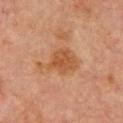Clinical impression:
Captured during whole-body skin photography for melanoma surveillance; the lesion was not biopsied.
Acquisition and patient details:
The lesion-visualizer software estimated an area of roughly 11 mm², an eccentricity of roughly 0.75, and a symmetry-axis asymmetry near 0.45. It also reported an average lesion color of about L≈42 a*≈20 b*≈31 (CIELAB), about 7 CIELAB-L* units darker than the surrounding skin, and a normalized border contrast of about 7. It also reported a classifier nevus-likeness of about 70/100 and lesion-presence confidence of about 100/100. The recorded lesion diameter is about 5.5 mm. The lesion is on the upper back. Cropped from a whole-body photographic skin survey; the tile spans about 15 mm. A male subject aged approximately 65. Imaged with cross-polarized lighting.